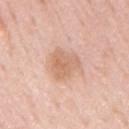This lesion was catalogued during total-body skin photography and was not selected for biopsy. The lesion is on the mid back. The lesion-visualizer software estimated a lesion area of about 9 mm², an outline eccentricity of about 0.55 (0 = round, 1 = elongated), and a shape-asymmetry score of about 0.35 (0 = symmetric). And it measured a color-variation rating of about 3/10 and a peripheral color-asymmetry measure near 1. A region of skin cropped from a whole-body photographic capture, roughly 15 mm wide. The patient is a male in their 60s.